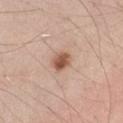Assessment:
No biopsy was performed on this lesion — it was imaged during a full skin examination and was not determined to be concerning.
Context:
This is a white-light tile. About 2.5 mm across. This image is a 15 mm lesion crop taken from a total-body photograph. The lesion-visualizer software estimated a mean CIELAB color near L≈55 a*≈21 b*≈30, roughly 14 lightness units darker than nearby skin, and a normalized border contrast of about 9.5. The software also gave border irregularity of about 1.5 on a 0–10 scale, a within-lesion color-variation index near 4/10, and radial color variation of about 1.5. On the left thigh. A male patient in their mid- to late 40s.